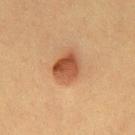– follow-up: no biopsy performed (imaged during a skin exam)
– diameter: ≈4 mm
– image: total-body-photography crop, ~15 mm field of view
– patient: female, roughly 40 years of age
– tile lighting: cross-polarized
– location: the mid back